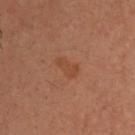Captured during whole-body skin photography for melanoma surveillance; the lesion was not biopsied.
A 15 mm close-up extracted from a 3D total-body photography capture.
A male subject approximately 40 years of age.
The lesion is on the head or neck.